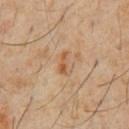The lesion was tiled from a total-body skin photograph and was not biopsied. A 15 mm close-up extracted from a 3D total-body photography capture. A male subject aged 58–62. The tile uses cross-polarized illumination. Approximately 3 mm at its widest. The lesion is located on the front of the torso. The total-body-photography lesion software estimated a border-irregularity index near 4.5/10, internal color variation of about 2 on a 0–10 scale, and peripheral color asymmetry of about 0.5. The software also gave a classifier nevus-likeness of about 0/100 and a lesion-detection confidence of about 100/100.